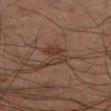Findings:
- acquisition — 15 mm crop, total-body photography
- anatomic site — the right forearm
- patient — male, roughly 70 years of age